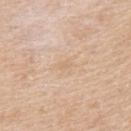The lesion was tiled from a total-body skin photograph and was not biopsied.
The lesion is located on the upper back.
An algorithmic analysis of the crop reported a footprint of about 1.5 mm² and a shape eccentricity near 0.8. The software also gave a mean CIELAB color near L≈69 a*≈16 b*≈34, roughly 5 lightness units darker than nearby skin, and a normalized lesion–skin contrast near 4. The software also gave a border-irregularity index near 2.5/10, a within-lesion color-variation index near 0/10, and a peripheral color-asymmetry measure near 0. It also reported a nevus-likeness score of about 0/100 and a detector confidence of about 100 out of 100 that the crop contains a lesion.
About 1.5 mm across.
A male patient, in their 60s.
The tile uses white-light illumination.
A 15 mm close-up tile from a total-body photography series done for melanoma screening.